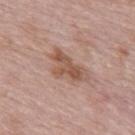Impression: Imaged during a routine full-body skin examination; the lesion was not biopsied and no histopathology is available. Background: Measured at roughly 5 mm in maximum diameter. Cropped from a whole-body photographic skin survey; the tile spans about 15 mm. The subject is a female aged around 65. The lesion is located on the upper back. Imaged with white-light lighting.